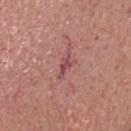biopsy_status: not biopsied; imaged during a skin examination
image:
  source: total-body photography crop
  field_of_view_mm: 15
patient:
  sex: male
  age_approx: 80
site: chest
lesion_size:
  long_diameter_mm_approx: 3.5
lighting: white-light